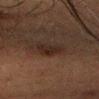Clinical impression: This lesion was catalogued during total-body skin photography and was not selected for biopsy. Context: Located on the head or neck. The lesion's longest dimension is about 4 mm. Automated image analysis of the tile measured an automated nevus-likeness rating near 65 out of 100 and a detector confidence of about 95 out of 100 that the crop contains a lesion. A female subject, approximately 55 years of age. A lesion tile, about 15 mm wide, cut from a 3D total-body photograph. The tile uses cross-polarized illumination.